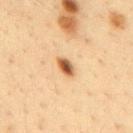Clinical impression:
This lesion was catalogued during total-body skin photography and was not selected for biopsy.
Context:
Longest diameter approximately 3 mm. Captured under cross-polarized illumination. A male subject, in their mid-30s. A region of skin cropped from a whole-body photographic capture, roughly 15 mm wide. The lesion is located on the mid back.